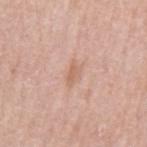The lesion was tiled from a total-body skin photograph and was not biopsied.
Automated image analysis of the tile measured an area of roughly 3 mm² and a shape-asymmetry score of about 0.4 (0 = symmetric). It also reported roughly 8 lightness units darker than nearby skin and a normalized border contrast of about 5.5. The analysis additionally found a border-irregularity rating of about 4/10, internal color variation of about 1.5 on a 0–10 scale, and a peripheral color-asymmetry measure near 0.5. It also reported a classifier nevus-likeness of about 0/100 and a detector confidence of about 100 out of 100 that the crop contains a lesion.
Longest diameter approximately 3 mm.
The lesion is located on the left upper arm.
The subject is a male aged 58 to 62.
Captured under white-light illumination.
A roughly 15 mm field-of-view crop from a total-body skin photograph.